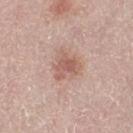Notes:
* biopsy status: catalogued during a skin exam; not biopsied
* image: total-body-photography crop, ~15 mm field of view
* patient: female, aged 63–67
* size: ~3.5 mm (longest diameter)
* tile lighting: white-light illumination
* location: the leg